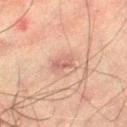Clinical summary:
A male subject in their 60s. The lesion-visualizer software estimated an outline eccentricity of about 0.75 (0 = round, 1 = elongated) and two-axis asymmetry of about 0.25. The software also gave a border-irregularity index near 2.5/10 and internal color variation of about 2.5 on a 0–10 scale. The lesion is located on the left thigh. This image is a 15 mm lesion crop taken from a total-body photograph. The tile uses cross-polarized illumination.